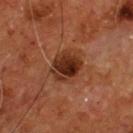The lesion was photographed on a routine skin check and not biopsied; there is no pathology result.
A male patient, in their mid- to late 50s.
Located on the chest.
Cropped from a whole-body photographic skin survey; the tile spans about 15 mm.